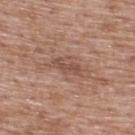{"biopsy_status": "not biopsied; imaged during a skin examination", "automated_metrics": {"border_irregularity_0_10": 7.0, "color_variation_0_10": 3.0, "peripheral_color_asymmetry": 1.0, "nevus_likeness_0_100": 0}, "lesion_size": {"long_diameter_mm_approx": 6.0}, "image": {"source": "total-body photography crop", "field_of_view_mm": 15}, "patient": {"sex": "male", "age_approx": 50}, "lighting": "white-light", "site": "upper back"}